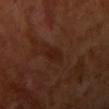| feature | finding |
|---|---|
| automated metrics | a lesion area of about 4 mm², an outline eccentricity of about 0.9 (0 = round, 1 = elongated), and a symmetry-axis asymmetry near 0.2; a within-lesion color-variation index near 1/10 and radial color variation of about 0.5; a nevus-likeness score of about 0/100 and a lesion-detection confidence of about 95/100 |
| lighting | cross-polarized |
| subject | female, aged approximately 55 |
| acquisition | ~15 mm crop, total-body skin-cancer survey |
| diameter | ≈3.5 mm |
| body site | the right upper arm |